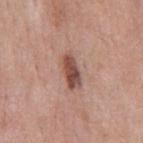Q: How was this image acquired?
A: ~15 mm tile from a whole-body skin photo
Q: Who is the patient?
A: male, roughly 55 years of age
Q: Lesion size?
A: ~4 mm (longest diameter)
Q: What is the anatomic site?
A: the chest
Q: What did automated image analysis measure?
A: an area of roughly 6.5 mm², an outline eccentricity of about 0.9 (0 = round, 1 = elongated), and a symmetry-axis asymmetry near 0.15; a mean CIELAB color near L≈49 a*≈21 b*≈25, a lesion–skin lightness drop of about 14, and a lesion-to-skin contrast of about 10 (normalized; higher = more distinct)